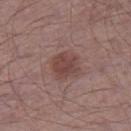  biopsy_status: not biopsied; imaged during a skin examination
  image:
    source: total-body photography crop
    field_of_view_mm: 15
  automated_metrics:
    area_mm2_approx: 8.5
    eccentricity: 0.2
    shape_asymmetry: 0.15
    border_irregularity_0_10: 1.5
    nevus_likeness_0_100: 15
  site: right thigh
  lighting: white-light
  lesion_size:
    long_diameter_mm_approx: 3.0
  patient:
    sex: male
    age_approx: 65